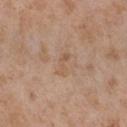{"site": "chest", "image": {"source": "total-body photography crop", "field_of_view_mm": 15}, "lesion_size": {"long_diameter_mm_approx": 3.0}, "patient": {"sex": "female", "age_approx": 65}}